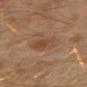notes: catalogued during a skin exam; not biopsied
location: the left arm
imaging modality: total-body-photography crop, ~15 mm field of view
automated lesion analysis: an area of roughly 11 mm², an outline eccentricity of about 0.8 (0 = round, 1 = elongated), and a symmetry-axis asymmetry near 0.3; an average lesion color of about L≈45 a*≈19 b*≈30 (CIELAB), a lesion–skin lightness drop of about 6, and a normalized border contrast of about 5; a border-irregularity rating of about 4/10, a color-variation rating of about 3.5/10, and radial color variation of about 1
patient: male, roughly 30 years of age